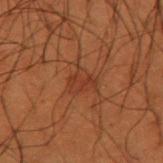Assessment: No biopsy was performed on this lesion — it was imaged during a full skin examination and was not determined to be concerning. Clinical summary: About 2.5 mm across. Imaged with cross-polarized lighting. The total-body-photography lesion software estimated an area of roughly 5 mm² and a symmetry-axis asymmetry near 0.3. A male patient, roughly 50 years of age. This image is a 15 mm lesion crop taken from a total-body photograph. From the left forearm.